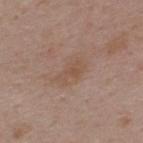The lesion was tiled from a total-body skin photograph and was not biopsied. The lesion is on the upper back. Measured at roughly 3.5 mm in maximum diameter. The tile uses white-light illumination. A female patient, aged approximately 45. A roughly 15 mm field-of-view crop from a total-body skin photograph.